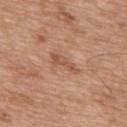– acquisition · 15 mm crop, total-body photography
– TBP lesion metrics · a lesion–skin lightness drop of about 8 and a normalized lesion–skin contrast near 6
– location · the mid back
– subject · male, aged around 50
– illumination · white-light illumination